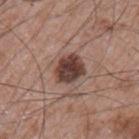follow-up — imaged on a skin check; not biopsied | site — the left upper arm | subject — male, roughly 65 years of age | lesion size — ≈4 mm | acquisition — total-body-photography crop, ~15 mm field of view | illumination — white-light illumination | automated lesion analysis — an outline eccentricity of about 0.6 (0 = round, 1 = elongated) and a shape-asymmetry score of about 0.2 (0 = symmetric); an average lesion color of about L≈40 a*≈19 b*≈22 (CIELAB).